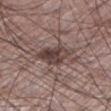Clinical impression: Part of a total-body skin-imaging series; this lesion was reviewed on a skin check and was not flagged for biopsy. Acquisition and patient details: This is a white-light tile. A male subject aged approximately 60. The recorded lesion diameter is about 6 mm. A 15 mm close-up tile from a total-body photography series done for melanoma screening. From the leg.